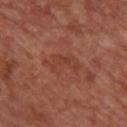The lesion was tiled from a total-body skin photograph and was not biopsied. The lesion is located on the upper back. Captured under cross-polarized illumination. A 15 mm close-up tile from a total-body photography series done for melanoma screening. The recorded lesion diameter is about 4.5 mm. A female subject, in their mid-40s.